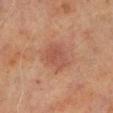biopsy_status: not biopsied; imaged during a skin examination
site: right lower leg
automated_metrics:
  cielab_L: 42
  cielab_a: 21
  cielab_b: 25
  vs_skin_darker_L: 6.0
  vs_skin_contrast_norm: 5.5
lighting: cross-polarized
patient:
  sex: male
  age_approx: 70
lesion_size:
  long_diameter_mm_approx: 4.5
image:
  source: total-body photography crop
  field_of_view_mm: 15